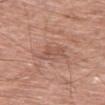Part of a total-body skin-imaging series; this lesion was reviewed on a skin check and was not flagged for biopsy.
A male patient approximately 60 years of age.
This is a white-light tile.
The lesion's longest dimension is about 3 mm.
A region of skin cropped from a whole-body photographic capture, roughly 15 mm wide.
Located on the left thigh.